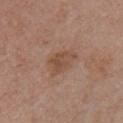Case summary:
• biopsy status — imaged on a skin check; not biopsied
• anatomic site — the chest
• image — ~15 mm tile from a whole-body skin photo
• subject — female, aged 53 to 57
• automated metrics — a border-irregularity index near 3.5/10 and a peripheral color-asymmetry measure near 1; an automated nevus-likeness rating near 0 out of 100 and a lesion-detection confidence of about 100/100
• illumination — white-light